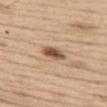Findings:
- anatomic site · the left thigh
- lighting · white-light
- acquisition · ~15 mm tile from a whole-body skin photo
- patient · male, aged 68 to 72
- size · ~3 mm (longest diameter)